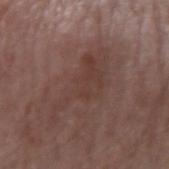Case summary:
* follow-up · imaged on a skin check; not biopsied
* image · 15 mm crop, total-body photography
* anatomic site · the left forearm
* patient · male, aged approximately 75